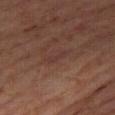biopsy status: total-body-photography surveillance lesion; no biopsy
patient: male, aged 58–62
lighting: cross-polarized illumination
anatomic site: the right thigh
lesion diameter: ~2.5 mm (longest diameter)
acquisition: ~15 mm crop, total-body skin-cancer survey
automated metrics: roughly 4 lightness units darker than nearby skin and a lesion-to-skin contrast of about 4.5 (normalized; higher = more distinct)